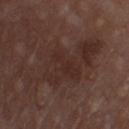The lesion was photographed on a routine skin check and not biopsied; there is no pathology result. This is a white-light tile. Longest diameter approximately 9.5 mm. A region of skin cropped from a whole-body photographic capture, roughly 15 mm wide. The lesion is located on the chest. The total-body-photography lesion software estimated an average lesion color of about L≈28 a*≈17 b*≈20 (CIELAB), about 6 CIELAB-L* units darker than the surrounding skin, and a normalized border contrast of about 6. And it measured a border-irregularity rating of about 8.5/10 and a color-variation rating of about 3/10. The software also gave a classifier nevus-likeness of about 0/100 and a lesion-detection confidence of about 95/100. The patient is a male aged 78 to 82.